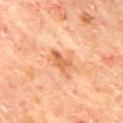<record>
  <biopsy_status>not biopsied; imaged during a skin examination</biopsy_status>
  <image>
    <source>total-body photography crop</source>
    <field_of_view_mm>15</field_of_view_mm>
  </image>
  <lesion_size>
    <long_diameter_mm_approx>3.0</long_diameter_mm_approx>
  </lesion_size>
  <patient>
    <sex>male</sex>
    <age_approx>65</age_approx>
  </patient>
  <site>mid back</site>
</record>